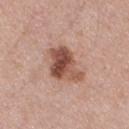The lesion is on the left upper arm. The patient is a male roughly 50 years of age. A roughly 15 mm field-of-view crop from a total-body skin photograph.